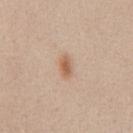notes = total-body-photography surveillance lesion; no biopsy
anatomic site = the front of the torso
automated metrics = a lesion color around L≈60 a*≈19 b*≈32 in CIELAB and roughly 10 lightness units darker than nearby skin; border irregularity of about 2.5 on a 0–10 scale and a peripheral color-asymmetry measure near 1.5
image = ~15 mm crop, total-body skin-cancer survey
subject = female, aged around 20
lesion size = ≈2.5 mm
illumination = white-light illumination